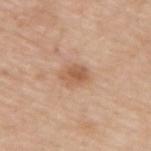This lesion was catalogued during total-body skin photography and was not selected for biopsy.
From the upper back.
The patient is a male aged approximately 65.
A roughly 15 mm field-of-view crop from a total-body skin photograph.
Imaged with white-light lighting.
Measured at roughly 3.5 mm in maximum diameter.